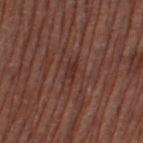Clinical impression:
This lesion was catalogued during total-body skin photography and was not selected for biopsy.
Background:
A male subject aged 73 to 77. Automated image analysis of the tile measured an average lesion color of about L≈31 a*≈21 b*≈22 (CIELAB), a lesion–skin lightness drop of about 7, and a normalized lesion–skin contrast near 6.5. And it measured border irregularity of about 4 on a 0–10 scale. About 2.5 mm across. Imaged with white-light lighting. Cropped from a whole-body photographic skin survey; the tile spans about 15 mm. On the left thigh.